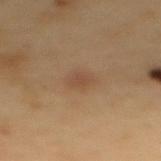workup = imaged on a skin check; not biopsied
image = 15 mm crop, total-body photography
anatomic site = the mid back
automated metrics = a lesion area of about 5.5 mm², a shape eccentricity near 0.75, and a symmetry-axis asymmetry near 0.2; a border-irregularity rating of about 2/10 and a color-variation rating of about 2/10
size = about 3 mm
tile lighting = cross-polarized illumination
patient = male, roughly 55 years of age